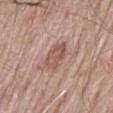Clinical impression: Captured during whole-body skin photography for melanoma surveillance; the lesion was not biopsied. Context: On the mid back. A male patient aged approximately 65. Captured under white-light illumination. Cropped from a whole-body photographic skin survey; the tile spans about 15 mm. Measured at roughly 4 mm in maximum diameter.